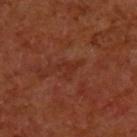No biopsy was performed on this lesion — it was imaged during a full skin examination and was not determined to be concerning.